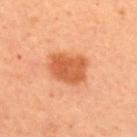{
  "biopsy_status": "not biopsied; imaged during a skin examination",
  "automated_metrics": {
    "border_irregularity_0_10": 2.0,
    "color_variation_0_10": 3.0,
    "peripheral_color_asymmetry": 1.0,
    "nevus_likeness_0_100": 100,
    "lesion_detection_confidence_0_100": 100
  },
  "image": {
    "source": "total-body photography crop",
    "field_of_view_mm": 15
  },
  "patient": {
    "sex": "female",
    "age_approx": 45
  },
  "site": "upper back",
  "lighting": "cross-polarized"
}